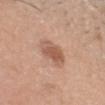The lesion was photographed on a routine skin check and not biopsied; there is no pathology result. The lesion is on the head or neck. A male patient, aged 28 to 32. Automated tile analysis of the lesion measured a lesion area of about 8.5 mm² and a symmetry-axis asymmetry near 0.15. The software also gave a normalized border contrast of about 7. And it measured a border-irregularity index near 2/10 and internal color variation of about 4 on a 0–10 scale. Cropped from a total-body skin-imaging series; the visible field is about 15 mm. Approximately 3.5 mm at its widest.